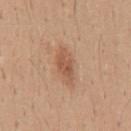Clinical impression:
The lesion was photographed on a routine skin check and not biopsied; there is no pathology result.
Background:
Captured under white-light illumination. A male subject, aged 38–42. A close-up tile cropped from a whole-body skin photograph, about 15 mm across. From the mid back.